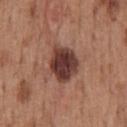This lesion was catalogued during total-body skin photography and was not selected for biopsy.
The lesion is on the back.
Approximately 4.5 mm at its widest.
A male subject, aged 58 to 62.
This image is a 15 mm lesion crop taken from a total-body photograph.
Imaged with white-light lighting.